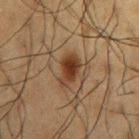No biopsy was performed on this lesion — it was imaged during a full skin examination and was not determined to be concerning.
Approximately 3.5 mm at its widest.
A male patient aged 58 to 62.
Automated tile analysis of the lesion measured a lesion color around L≈29 a*≈16 b*≈26 in CIELAB, a lesion–skin lightness drop of about 10, and a normalized border contrast of about 10.5. The analysis additionally found an automated nevus-likeness rating near 95 out of 100 and lesion-presence confidence of about 100/100.
A roughly 15 mm field-of-view crop from a total-body skin photograph.
From the right upper arm.
This is a cross-polarized tile.